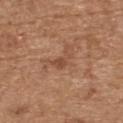About 3 mm across. A 15 mm close-up extracted from a 3D total-body photography capture. A male patient, in their 70s. Located on the upper back. This is a white-light tile.